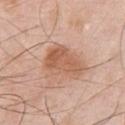| feature | finding |
|---|---|
| automated metrics | an area of roughly 13 mm² and an outline eccentricity of about 0.8 (0 = round, 1 = elongated); border irregularity of about 3.5 on a 0–10 scale, a within-lesion color-variation index near 4/10, and peripheral color asymmetry of about 1; a nevus-likeness score of about 5/100 and a detector confidence of about 100 out of 100 that the crop contains a lesion |
| diameter | ≈5 mm |
| subject | male, aged 53–57 |
| body site | the chest |
| image source | 15 mm crop, total-body photography |
| illumination | white-light |